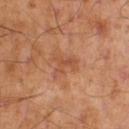Captured during whole-body skin photography for melanoma surveillance; the lesion was not biopsied. This is a cross-polarized tile. A male subject, in their mid-50s. The lesion's longest dimension is about 3 mm. Located on the right thigh. A 15 mm close-up tile from a total-body photography series done for melanoma screening.